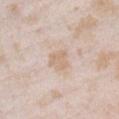Clinical impression:
The lesion was photographed on a routine skin check and not biopsied; there is no pathology result.